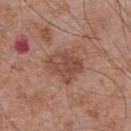* biopsy status: catalogued during a skin exam; not biopsied
* anatomic site: the abdomen
* lighting: white-light
* patient: male, roughly 70 years of age
* image source: ~15 mm crop, total-body skin-cancer survey
* lesion size: about 4 mm
* image-analysis metrics: an area of roughly 11 mm², an outline eccentricity of about 0.6 (0 = round, 1 = elongated), and a symmetry-axis asymmetry near 0.3; a mean CIELAB color near L≈47 a*≈22 b*≈27, a lesion–skin lightness drop of about 9, and a lesion-to-skin contrast of about 6.5 (normalized; higher = more distinct); a border-irregularity index near 3/10, a color-variation rating of about 4/10, and radial color variation of about 1.5; a classifier nevus-likeness of about 0/100 and a lesion-detection confidence of about 100/100